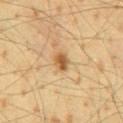Impression: The lesion was tiled from a total-body skin photograph and was not biopsied. Context: A 15 mm close-up tile from a total-body photography series done for melanoma screening. The subject is a male aged around 65. On the lower back.